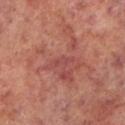Q: Was this lesion biopsied?
A: catalogued during a skin exam; not biopsied
Q: What is the anatomic site?
A: the left lower leg
Q: What kind of image is this?
A: 15 mm crop, total-body photography
Q: What lighting was used for the tile?
A: cross-polarized
Q: What are the patient's age and sex?
A: male, aged 63 to 67
Q: Automated lesion metrics?
A: roughly 6 lightness units darker than nearby skin and a normalized border contrast of about 5; an automated nevus-likeness rating near 0 out of 100 and lesion-presence confidence of about 95/100
Q: How large is the lesion?
A: ~3 mm (longest diameter)